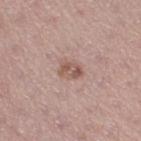The lesion was photographed on a routine skin check and not biopsied; there is no pathology result.
The lesion is on the leg.
A 15 mm close-up tile from a total-body photography series done for melanoma screening.
A female patient approximately 40 years of age.
About 2.5 mm across.